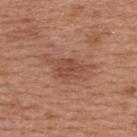lesion diameter — about 6 mm; patient — male, aged around 30; image — 15 mm crop, total-body photography; illumination — white-light illumination; anatomic site — the upper back.